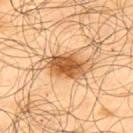The lesion was tiled from a total-body skin photograph and was not biopsied.
This image is a 15 mm lesion crop taken from a total-body photograph.
A male subject, approximately 65 years of age.
Approximately 4.5 mm at its widest.
The tile uses cross-polarized illumination.
The lesion is located on the upper back.
The total-body-photography lesion software estimated an average lesion color of about L≈54 a*≈23 b*≈41 (CIELAB), about 16 CIELAB-L* units darker than the surrounding skin, and a normalized border contrast of about 11. And it measured internal color variation of about 4.5 on a 0–10 scale and a peripheral color-asymmetry measure near 1.5. It also reported a nevus-likeness score of about 95/100 and a detector confidence of about 100 out of 100 that the crop contains a lesion.